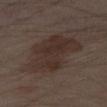Q: Was this lesion biopsied?
A: total-body-photography surveillance lesion; no biopsy
Q: Where on the body is the lesion?
A: the right thigh
Q: Lesion size?
A: ≈7.5 mm
Q: Illumination type?
A: cross-polarized illumination
Q: How was this image acquired?
A: ~15 mm crop, total-body skin-cancer survey
Q: Patient demographics?
A: aged 53 to 57